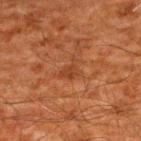Impression: This lesion was catalogued during total-body skin photography and was not selected for biopsy. Clinical summary: Imaged with cross-polarized lighting. The subject is a male aged approximately 60. On the back. A lesion tile, about 15 mm wide, cut from a 3D total-body photograph. The recorded lesion diameter is about 3 mm.